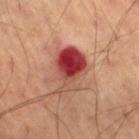Part of a total-body skin-imaging series; this lesion was reviewed on a skin check and was not flagged for biopsy.
A close-up tile cropped from a whole-body skin photograph, about 15 mm across.
Automated image analysis of the tile measured an average lesion color of about L≈43 a*≈32 b*≈27 (CIELAB) and about 16 CIELAB-L* units darker than the surrounding skin. The software also gave a border-irregularity index near 4.5/10, a within-lesion color-variation index near 10/10, and radial color variation of about 4.5. It also reported a classifier nevus-likeness of about 0/100 and a detector confidence of about 100 out of 100 that the crop contains a lesion.
A male subject, approximately 70 years of age.
This is a cross-polarized tile.
On the right thigh.
The recorded lesion diameter is about 5.5 mm.